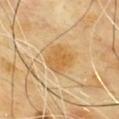Part of a total-body skin-imaging series; this lesion was reviewed on a skin check and was not flagged for biopsy. A male subject, approximately 65 years of age. On the chest. A region of skin cropped from a whole-body photographic capture, roughly 15 mm wide. Measured at roughly 4 mm in maximum diameter. An algorithmic analysis of the crop reported a shape eccentricity near 0.55 and two-axis asymmetry of about 0.15. And it measured a border-irregularity rating of about 1.5/10. The software also gave an automated nevus-likeness rating near 80 out of 100 and lesion-presence confidence of about 100/100.